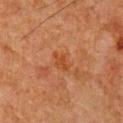| field | value |
|---|---|
| notes | total-body-photography surveillance lesion; no biopsy |
| patient | male, in their 80s |
| automated metrics | a footprint of about 3 mm²; a color-variation rating of about 0/10; a classifier nevus-likeness of about 0/100 and lesion-presence confidence of about 100/100 |
| anatomic site | the chest |
| image source | ~15 mm crop, total-body skin-cancer survey |
| illumination | cross-polarized |
| lesion diameter | ≈2.5 mm |